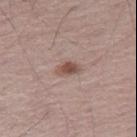Impression: No biopsy was performed on this lesion — it was imaged during a full skin examination and was not determined to be concerning. Image and clinical context: From the right thigh. The recorded lesion diameter is about 2.5 mm. A male subject, in their 60s. This is a white-light tile. A close-up tile cropped from a whole-body skin photograph, about 15 mm across.